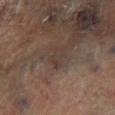Q: Was a biopsy performed?
A: imaged on a skin check; not biopsied
Q: Where on the body is the lesion?
A: the left lower leg
Q: What is the imaging modality?
A: ~15 mm crop, total-body skin-cancer survey
Q: Who is the patient?
A: male, aged around 65
Q: How was the tile lit?
A: cross-polarized
Q: What is the lesion's diameter?
A: ~2.5 mm (longest diameter)